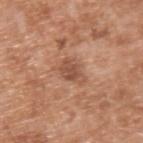Findings:
– biopsy status — imaged on a skin check; not biopsied
– automated lesion analysis — a lesion area of about 5.5 mm²; an average lesion color of about L≈51 a*≈23 b*≈31 (CIELAB), about 9 CIELAB-L* units darker than the surrounding skin, and a lesion-to-skin contrast of about 6.5 (normalized; higher = more distinct)
– size — about 3 mm
– image — ~15 mm crop, total-body skin-cancer survey
– site — the upper back
– subject — male, aged 63 to 67
– tile lighting — white-light